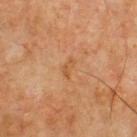This lesion was catalogued during total-body skin photography and was not selected for biopsy. Located on the front of the torso. An algorithmic analysis of the crop reported an average lesion color of about L≈47 a*≈20 b*≈35 (CIELAB), roughly 5 lightness units darker than nearby skin, and a normalized border contrast of about 5. It also reported a border-irregularity index near 4/10, internal color variation of about 1.5 on a 0–10 scale, and a peripheral color-asymmetry measure near 0.5. Captured under cross-polarized illumination. A roughly 15 mm field-of-view crop from a total-body skin photograph. A male patient, roughly 65 years of age.